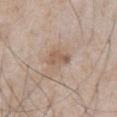Q: What is the imaging modality?
A: ~15 mm tile from a whole-body skin photo
Q: Where on the body is the lesion?
A: the abdomen
Q: Lesion size?
A: about 2.5 mm
Q: What lighting was used for the tile?
A: white-light illumination
Q: What are the patient's age and sex?
A: male, in their mid-60s